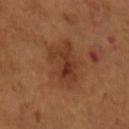This lesion was catalogued during total-body skin photography and was not selected for biopsy. The recorded lesion diameter is about 6 mm. The lesion is on the right forearm. The tile uses cross-polarized illumination. Automated image analysis of the tile measured a shape eccentricity near 0.75. And it measured border irregularity of about 3.5 on a 0–10 scale and a peripheral color-asymmetry measure near 2. The analysis additionally found a classifier nevus-likeness of about 0/100 and a lesion-detection confidence of about 100/100. A region of skin cropped from a whole-body photographic capture, roughly 15 mm wide. A female subject roughly 55 years of age.